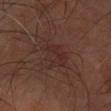Acquisition and patient details:
The patient is a male in their mid- to late 60s. Located on the left forearm. Cropped from a total-body skin-imaging series; the visible field is about 15 mm.